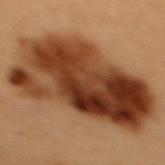The lesion was biopsied, and histopathology showed a dysplastic (Clark) nevus.